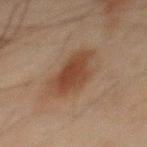Clinical impression:
The lesion was photographed on a routine skin check and not biopsied; there is no pathology result.
Clinical summary:
The subject is a male aged around 45. A 15 mm close-up extracted from a 3D total-body photography capture. Imaged with cross-polarized lighting. An algorithmic analysis of the crop reported an area of roughly 15 mm², a shape eccentricity near 0.85, and a symmetry-axis asymmetry near 0.2. It also reported a lesion color around L≈35 a*≈17 b*≈25 in CIELAB and a lesion–skin lightness drop of about 9. The software also gave a within-lesion color-variation index near 3.5/10 and radial color variation of about 1. The software also gave a classifier nevus-likeness of about 100/100 and a lesion-detection confidence of about 100/100. On the mid back.